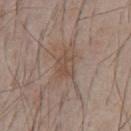Assessment:
This lesion was catalogued during total-body skin photography and was not selected for biopsy.
Background:
The recorded lesion diameter is about 3.5 mm. The patient is a male in their mid-60s. A close-up tile cropped from a whole-body skin photograph, about 15 mm across. The lesion-visualizer software estimated an average lesion color of about L≈49 a*≈15 b*≈26 (CIELAB), about 7 CIELAB-L* units darker than the surrounding skin, and a lesion-to-skin contrast of about 5.5 (normalized; higher = more distinct). And it measured a border-irregularity rating of about 3.5/10 and radial color variation of about 1. Imaged with white-light lighting. On the chest.